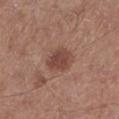This lesion was catalogued during total-body skin photography and was not selected for biopsy.
Captured under white-light illumination.
A male patient aged around 60.
A close-up tile cropped from a whole-body skin photograph, about 15 mm across.
On the left lower leg.